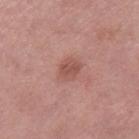The lesion was tiled from a total-body skin photograph and was not biopsied. On the leg. The subject is a female approximately 70 years of age. A close-up tile cropped from a whole-body skin photograph, about 15 mm across.